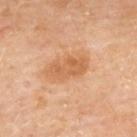Impression:
Imaged during a routine full-body skin examination; the lesion was not biopsied and no histopathology is available.
Acquisition and patient details:
Captured under cross-polarized illumination. Automated tile analysis of the lesion measured a footprint of about 8.5 mm² and two-axis asymmetry of about 0.25. The software also gave an average lesion color of about L≈61 a*≈24 b*≈39 (CIELAB), a lesion–skin lightness drop of about 9, and a lesion-to-skin contrast of about 6.5 (normalized; higher = more distinct). The software also gave border irregularity of about 3 on a 0–10 scale, a within-lesion color-variation index near 2.5/10, and peripheral color asymmetry of about 1. And it measured a nevus-likeness score of about 10/100 and lesion-presence confidence of about 100/100. A female subject aged 58–62. Located on the upper back. About 4.5 mm across. A roughly 15 mm field-of-view crop from a total-body skin photograph.